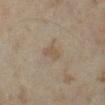Q: Was this lesion biopsied?
A: no biopsy performed (imaged during a skin exam)
Q: What kind of image is this?
A: ~15 mm tile from a whole-body skin photo
Q: How was the tile lit?
A: cross-polarized
Q: What did automated image analysis measure?
A: a footprint of about 3 mm², an outline eccentricity of about 0.8 (0 = round, 1 = elongated), and a symmetry-axis asymmetry near 0.35
Q: What are the patient's age and sex?
A: female, aged around 35
Q: Where on the body is the lesion?
A: the leg
Q: What is the lesion's diameter?
A: ~2.5 mm (longest diameter)